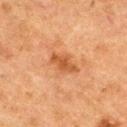biopsy status: imaged on a skin check; not biopsied
site: the upper back
tile lighting: cross-polarized
patient: female, about 60 years old
image source: ~15 mm crop, total-body skin-cancer survey